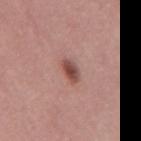Q: Was a biopsy performed?
A: total-body-photography surveillance lesion; no biopsy
Q: What is the anatomic site?
A: the mid back
Q: What is the imaging modality?
A: total-body-photography crop, ~15 mm field of view
Q: Lesion size?
A: ≈3 mm
Q: What are the patient's age and sex?
A: male, approximately 40 years of age